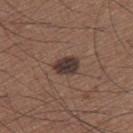Imaged with white-light lighting.
The lesion's longest dimension is about 3 mm.
The subject is a male aged 63–67.
A 15 mm close-up tile from a total-body photography series done for melanoma screening.
The lesion is on the right thigh.